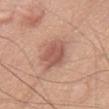Q: Is there a histopathology result?
A: no biopsy performed (imaged during a skin exam)